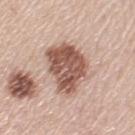| key | value |
|---|---|
| follow-up | total-body-photography surveillance lesion; no biopsy |
| patient | female, aged approximately 60 |
| illumination | white-light illumination |
| lesion size | about 6.5 mm |
| site | the left upper arm |
| image source | ~15 mm crop, total-body skin-cancer survey |